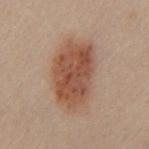The lesion was tiled from a total-body skin photograph and was not biopsied.
From the front of the torso.
Measured at roughly 8 mm in maximum diameter.
The lesion-visualizer software estimated a lesion area of about 27 mm², a shape eccentricity near 0.85, and a symmetry-axis asymmetry near 0.2.
A region of skin cropped from a whole-body photographic capture, roughly 15 mm wide.
Imaged with cross-polarized lighting.
The patient is a male in their 50s.